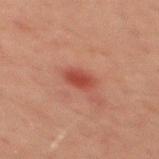Q: Lesion location?
A: the mid back
Q: Patient demographics?
A: male, in their mid- to late 40s
Q: What is the lesion's diameter?
A: ~2.5 mm (longest diameter)
Q: What kind of image is this?
A: ~15 mm tile from a whole-body skin photo